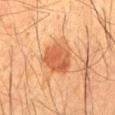Imaged during a routine full-body skin examination; the lesion was not biopsied and no histopathology is available. This is a cross-polarized tile. A male patient in their mid-30s. A region of skin cropped from a whole-body photographic capture, roughly 15 mm wide. The recorded lesion diameter is about 4.5 mm. Automated tile analysis of the lesion measured a symmetry-axis asymmetry near 0.2. And it measured an average lesion color of about L≈46 a*≈23 b*≈33 (CIELAB), a lesion–skin lightness drop of about 10, and a lesion-to-skin contrast of about 7.5 (normalized; higher = more distinct). And it measured a border-irregularity index near 2/10, internal color variation of about 3.5 on a 0–10 scale, and a peripheral color-asymmetry measure near 1.5. The software also gave a classifier nevus-likeness of about 95/100 and a detector confidence of about 100 out of 100 that the crop contains a lesion. From the mid back.